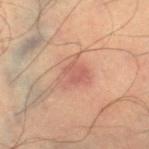This lesion was catalogued during total-body skin photography and was not selected for biopsy.
A 15 mm close-up tile from a total-body photography series done for melanoma screening.
Longest diameter approximately 3.5 mm.
Located on the leg.
The subject is a male in their mid- to late 30s.